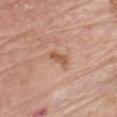Assessment:
The lesion was tiled from a total-body skin photograph and was not biopsied.
Image and clinical context:
The subject is a male aged around 80. The total-body-photography lesion software estimated an outline eccentricity of about 0.85 (0 = round, 1 = elongated) and two-axis asymmetry of about 0.3. It also reported a lesion color around L≈56 a*≈22 b*≈33 in CIELAB and a normalized lesion–skin contrast near 7.5. The software also gave border irregularity of about 3 on a 0–10 scale. And it measured an automated nevus-likeness rating near 10 out of 100 and a detector confidence of about 100 out of 100 that the crop contains a lesion. From the chest. Imaged with white-light lighting. This image is a 15 mm lesion crop taken from a total-body photograph.